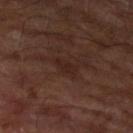Assessment:
Part of a total-body skin-imaging series; this lesion was reviewed on a skin check and was not flagged for biopsy.